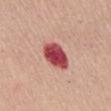Q: Was a biopsy performed?
A: imaged on a skin check; not biopsied
Q: Patient demographics?
A: female, aged 53 to 57
Q: Lesion location?
A: the mid back
Q: What did automated image analysis measure?
A: a footprint of about 10 mm²; a border-irregularity rating of about 1.5/10, internal color variation of about 5.5 on a 0–10 scale, and a peripheral color-asymmetry measure near 1.5
Q: Lesion size?
A: ≈4 mm
Q: What kind of image is this?
A: ~15 mm tile from a whole-body skin photo
Q: What lighting was used for the tile?
A: white-light